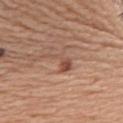This lesion was catalogued during total-body skin photography and was not selected for biopsy. A 15 mm close-up extracted from a 3D total-body photography capture. The tile uses white-light illumination. On the upper back. An algorithmic analysis of the crop reported a mean CIELAB color near L≈51 a*≈22 b*≈29. The software also gave a classifier nevus-likeness of about 50/100. The recorded lesion diameter is about 4 mm. A male subject, aged approximately 65.